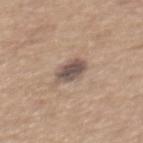Part of a total-body skin-imaging series; this lesion was reviewed on a skin check and was not flagged for biopsy.